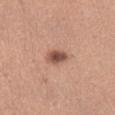Assessment:
Part of a total-body skin-imaging series; this lesion was reviewed on a skin check and was not flagged for biopsy.
Acquisition and patient details:
The patient is a female roughly 55 years of age. On the front of the torso. Imaged with white-light lighting. A 15 mm close-up extracted from a 3D total-body photography capture. The lesion's longest dimension is about 3 mm. The lesion-visualizer software estimated an eccentricity of roughly 0.75 and two-axis asymmetry of about 0.25. And it measured a border-irregularity rating of about 2/10, internal color variation of about 4.5 on a 0–10 scale, and radial color variation of about 1.5.